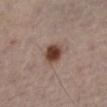Assessment: Captured during whole-body skin photography for melanoma surveillance; the lesion was not biopsied. Context: Cropped from a total-body skin-imaging series; the visible field is about 15 mm. The lesion's longest dimension is about 3 mm. The subject is a male in their 50s. The lesion is on the leg. This is a cross-polarized tile.